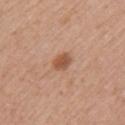Imaged with white-light lighting.
A female patient, in their 30s.
The lesion is located on the left upper arm.
A roughly 15 mm field-of-view crop from a total-body skin photograph.